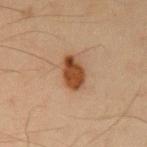Clinical impression:
No biopsy was performed on this lesion — it was imaged during a full skin examination and was not determined to be concerning.
Context:
A male subject in their mid-30s. An algorithmic analysis of the crop reported a shape eccentricity near 0.8 and a symmetry-axis asymmetry near 0.15. And it measured a nevus-likeness score of about 100/100 and a detector confidence of about 100 out of 100 that the crop contains a lesion. Located on the left upper arm. Cropped from a total-body skin-imaging series; the visible field is about 15 mm. The lesion's longest dimension is about 4 mm.